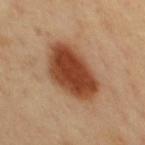follow-up: no biopsy performed (imaged during a skin exam)
image source: ~15 mm crop, total-body skin-cancer survey
anatomic site: the chest
patient: male, roughly 50 years of age
illumination: cross-polarized illumination
TBP lesion metrics: a lesion color around L≈44 a*≈25 b*≈34 in CIELAB; border irregularity of about 2 on a 0–10 scale and a color-variation rating of about 5/10